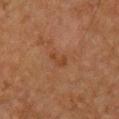Clinical impression:
Recorded during total-body skin imaging; not selected for excision or biopsy.
Background:
A male patient approximately 65 years of age. Approximately 2.5 mm at its widest. This is a cross-polarized tile. A 15 mm crop from a total-body photograph taken for skin-cancer surveillance. The lesion is located on the chest. The total-body-photography lesion software estimated a footprint of about 3 mm², an eccentricity of roughly 0.8, and a shape-asymmetry score of about 0.4 (0 = symmetric). It also reported a lesion-to-skin contrast of about 5.5 (normalized; higher = more distinct). The software also gave a border-irregularity rating of about 4.5/10 and a within-lesion color-variation index near 1/10. And it measured a lesion-detection confidence of about 100/100.